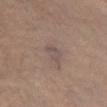notes = imaged on a skin check; not biopsied | automated lesion analysis = an average lesion color of about L≈48 a*≈14 b*≈19 (CIELAB) and about 6 CIELAB-L* units darker than the surrounding skin; a border-irregularity rating of about 3.5/10, a within-lesion color-variation index near 1.5/10, and a peripheral color-asymmetry measure near 0.5 | anatomic site = the left leg | lesion size = ≈2.5 mm | imaging modality = ~15 mm tile from a whole-body skin photo | subject = male, aged 48–52 | illumination = cross-polarized.